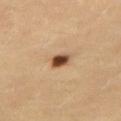size: ≈3 mm | image-analysis metrics: a footprint of about 4.5 mm², an eccentricity of roughly 0.75, and a symmetry-axis asymmetry near 0.2; an average lesion color of about L≈51 a*≈20 b*≈34 (CIELAB) and about 18 CIELAB-L* units darker than the surrounding skin; lesion-presence confidence of about 100/100 | patient: female, aged 68 to 72 | anatomic site: the right thigh | image source: ~15 mm crop, total-body skin-cancer survey | illumination: cross-polarized illumination.